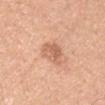Findings:
• workup: catalogued during a skin exam; not biopsied
• automated metrics: a lesion area of about 6 mm², a shape eccentricity near 0.6, and a symmetry-axis asymmetry near 0.3; a peripheral color-asymmetry measure near 1.5; lesion-presence confidence of about 100/100
• subject: male, in their 30s
• lesion diameter: ~3 mm (longest diameter)
• acquisition: 15 mm crop, total-body photography
• tile lighting: white-light illumination
• site: the right upper arm